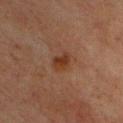Captured during whole-body skin photography for melanoma surveillance; the lesion was not biopsied.
The lesion is on the back.
A lesion tile, about 15 mm wide, cut from a 3D total-body photograph.
The recorded lesion diameter is about 2.5 mm.
Captured under cross-polarized illumination.
A male patient, about 65 years old.